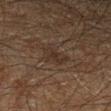Imaged during a routine full-body skin examination; the lesion was not biopsied and no histopathology is available.
The lesion is located on the leg.
A 15 mm close-up tile from a total-body photography series done for melanoma screening.
The lesion-visualizer software estimated border irregularity of about 4 on a 0–10 scale, a color-variation rating of about 1.5/10, and peripheral color asymmetry of about 0.5. The software also gave a nevus-likeness score of about 0/100 and a lesion-detection confidence of about 70/100.
Captured under cross-polarized illumination.
A male patient, about 60 years old.